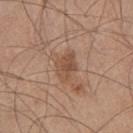{"biopsy_status": "not biopsied; imaged during a skin examination", "patient": {"sex": "male", "age_approx": 45}, "image": {"source": "total-body photography crop", "field_of_view_mm": 15}, "site": "leg", "lighting": "white-light", "lesion_size": {"long_diameter_mm_approx": 3.5}}